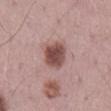Captured during whole-body skin photography for melanoma surveillance; the lesion was not biopsied.
Measured at roughly 4 mm in maximum diameter.
Cropped from a total-body skin-imaging series; the visible field is about 15 mm.
On the mid back.
A male subject, aged 68 to 72.
Captured under white-light illumination.
Automated image analysis of the tile measured a classifier nevus-likeness of about 95/100 and a detector confidence of about 100 out of 100 that the crop contains a lesion.